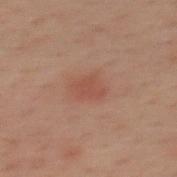Findings:
• biopsy status — imaged on a skin check; not biopsied
• tile lighting — cross-polarized illumination
• lesion diameter — about 3 mm
• acquisition — 15 mm crop, total-body photography
• body site — the back
• subject — male, in their 40s